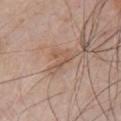Recorded during total-body skin imaging; not selected for excision or biopsy.
On the chest.
A lesion tile, about 15 mm wide, cut from a 3D total-body photograph.
Approximately 4 mm at its widest.
A male subject, aged approximately 55.